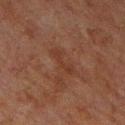Notes:
* notes: catalogued during a skin exam; not biopsied
* size: ≈4 mm
* image: ~15 mm crop, total-body skin-cancer survey
* subject: male, about 60 years old
* tile lighting: cross-polarized
* anatomic site: the chest